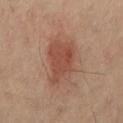acquisition — total-body-photography crop, ~15 mm field of view
anatomic site — the back
lighting — cross-polarized
patient — male, approximately 60 years of age
image-analysis metrics — a mean CIELAB color near L≈44 a*≈21 b*≈27, a lesion–skin lightness drop of about 9, and a lesion-to-skin contrast of about 7 (normalized; higher = more distinct); a classifier nevus-likeness of about 100/100